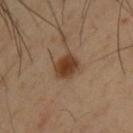The lesion was tiled from a total-body skin photograph and was not biopsied.
Imaged with cross-polarized lighting.
A male subject, about 40 years old.
About 3 mm across.
A region of skin cropped from a whole-body photographic capture, roughly 15 mm wide.
The lesion is located on the upper back.
An algorithmic analysis of the crop reported a color-variation rating of about 2.5/10 and a peripheral color-asymmetry measure near 0.5. The analysis additionally found an automated nevus-likeness rating near 100 out of 100 and lesion-presence confidence of about 100/100.